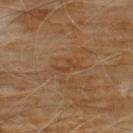Clinical impression: Recorded during total-body skin imaging; not selected for excision or biopsy. Acquisition and patient details: Longest diameter approximately 2.5 mm. A male subject aged around 60. Imaged with cross-polarized lighting. On the chest. A 15 mm close-up extracted from a 3D total-body photography capture.